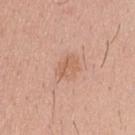follow-up: no biopsy performed (imaged during a skin exam)
imaging modality: ~15 mm tile from a whole-body skin photo
automated metrics: a border-irregularity index near 3/10, internal color variation of about 2.5 on a 0–10 scale, and a peripheral color-asymmetry measure near 1; an automated nevus-likeness rating near 5 out of 100 and a lesion-detection confidence of about 100/100
site: the upper back
patient: male, in their 40s
illumination: white-light